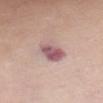Context: Located on the chest. The subject is a female aged approximately 65. Cropped from a whole-body photographic skin survey; the tile spans about 15 mm. The tile uses white-light illumination. Automated tile analysis of the lesion measured a footprint of about 8.5 mm², an outline eccentricity of about 0.8 (0 = round, 1 = elongated), and a symmetry-axis asymmetry near 0.25. The software also gave an average lesion color of about L≈57 a*≈22 b*≈18 (CIELAB) and a lesion–skin lightness drop of about 13. The analysis additionally found an automated nevus-likeness rating near 0 out of 100. Measured at roughly 4 mm in maximum diameter.